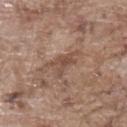Impression: Recorded during total-body skin imaging; not selected for excision or biopsy. Acquisition and patient details: On the upper back. This image is a 15 mm lesion crop taken from a total-body photograph. Approximately 3 mm at its widest. This is a white-light tile. A male patient, about 70 years old. The lesion-visualizer software estimated a lesion-detection confidence of about 85/100.